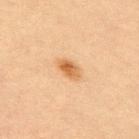{
  "biopsy_status": "not biopsied; imaged during a skin examination"
}